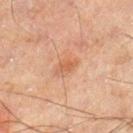The lesion was tiled from a total-body skin photograph and was not biopsied.
A 15 mm close-up tile from a total-body photography series done for melanoma screening.
Captured under cross-polarized illumination.
The lesion is located on the right lower leg.
The subject is a male aged approximately 45.
The recorded lesion diameter is about 2.5 mm.